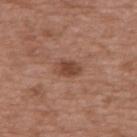No biopsy was performed on this lesion — it was imaged during a full skin examination and was not determined to be concerning. A 15 mm close-up extracted from a 3D total-body photography capture. A female subject in their mid- to late 70s. Imaged with white-light lighting. Longest diameter approximately 3 mm. Located on the mid back.